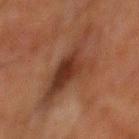Recorded during total-body skin imaging; not selected for excision or biopsy. Longest diameter approximately 8.5 mm. A male subject about 80 years old. Cropped from a whole-body photographic skin survey; the tile spans about 15 mm. Captured under cross-polarized illumination. From the mid back. An algorithmic analysis of the crop reported an average lesion color of about L≈29 a*≈18 b*≈24 (CIELAB) and roughly 8 lightness units darker than nearby skin. The analysis additionally found a border-irregularity index near 7/10, a within-lesion color-variation index near 5.5/10, and a peripheral color-asymmetry measure near 2.